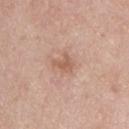Recorded during total-body skin imaging; not selected for excision or biopsy.